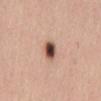  biopsy_status: not biopsied; imaged during a skin examination
  patient:
    sex: female
    age_approx: 55
  lesion_size:
    long_diameter_mm_approx: 3.0
  image:
    source: total-body photography crop
    field_of_view_mm: 15
  site: lower back